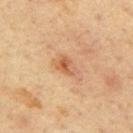  biopsy_status: not biopsied; imaged during a skin examination
  lesion_size:
    long_diameter_mm_approx: 3.0
  image:
    source: total-body photography crop
    field_of_view_mm: 15
  patient:
    sex: male
    age_approx: 65
  site: mid back